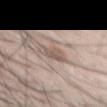patient: male, roughly 70 years of age; site: the chest; TBP lesion metrics: a lesion area of about 5 mm², a shape eccentricity near 0.6, and a shape-asymmetry score of about 0.25 (0 = symmetric); imaging modality: ~15 mm tile from a whole-body skin photo; lighting: white-light; diameter: about 2.5 mm.